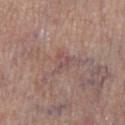Assessment:
Recorded during total-body skin imaging; not selected for excision or biopsy.
Acquisition and patient details:
This image is a 15 mm lesion crop taken from a total-body photograph. Automated tile analysis of the lesion measured a lesion color around L≈50 a*≈20 b*≈20 in CIELAB, a lesion–skin lightness drop of about 6, and a lesion-to-skin contrast of about 5 (normalized; higher = more distinct). It also reported a classifier nevus-likeness of about 0/100 and lesion-presence confidence of about 55/100. Imaged with white-light lighting. Measured at roughly 2.5 mm in maximum diameter. The subject is a male roughly 70 years of age. The lesion is located on the left lower leg.